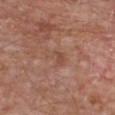Acquisition and patient details:
The lesion is on the chest. The subject is a male roughly 65 years of age. About 3 mm across. A lesion tile, about 15 mm wide, cut from a 3D total-body photograph.